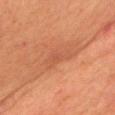<case>
<biopsy_status>not biopsied; imaged during a skin examination</biopsy_status>
<image>
  <source>total-body photography crop</source>
  <field_of_view_mm>15</field_of_view_mm>
</image>
<patient>
  <sex>female</sex>
  <age_approx>55</age_approx>
</patient>
<site>chest</site>
</case>